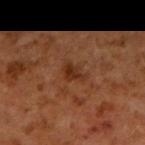<lesion>
  <biopsy_status>not biopsied; imaged during a skin examination</biopsy_status>
  <site>right upper arm</site>
  <lighting>cross-polarized</lighting>
  <lesion_size>
    <long_diameter_mm_approx>2.5</long_diameter_mm_approx>
  </lesion_size>
  <image>
    <source>total-body photography crop</source>
    <field_of_view_mm>15</field_of_view_mm>
  </image>
  <patient>
    <sex>male</sex>
    <age_approx>60</age_approx>
  </patient>
</lesion>